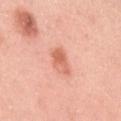{
  "biopsy_status": "not biopsied; imaged during a skin examination",
  "lighting": "white-light",
  "patient": {
    "sex": "female",
    "age_approx": 25
  },
  "automated_metrics": {
    "cielab_L": 65,
    "cielab_a": 30,
    "cielab_b": 34,
    "vs_skin_darker_L": 11.0,
    "vs_skin_contrast_norm": 7.0,
    "nevus_likeness_0_100": 60,
    "lesion_detection_confidence_0_100": 100
  },
  "image": {
    "source": "total-body photography crop",
    "field_of_view_mm": 15
  },
  "site": "left upper arm"
}